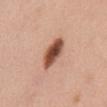Q: Is there a histopathology result?
A: imaged on a skin check; not biopsied
Q: Lesion location?
A: the front of the torso
Q: What is the imaging modality?
A: ~15 mm tile from a whole-body skin photo
Q: Patient demographics?
A: female, approximately 50 years of age
Q: What lighting was used for the tile?
A: white-light illumination